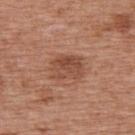Case summary:
- notes: no biopsy performed (imaged during a skin exam)
- image-analysis metrics: a lesion color around L≈48 a*≈23 b*≈30 in CIELAB, roughly 9 lightness units darker than nearby skin, and a normalized lesion–skin contrast near 6.5
- acquisition: ~15 mm crop, total-body skin-cancer survey
- patient: female, roughly 40 years of age
- location: the upper back
- lesion diameter: ~4 mm (longest diameter)
- illumination: white-light illumination